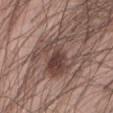Q: Is there a histopathology result?
A: catalogued during a skin exam; not biopsied
Q: Where on the body is the lesion?
A: the abdomen
Q: How was the tile lit?
A: white-light illumination
Q: Patient demographics?
A: male, about 55 years old
Q: How was this image acquired?
A: total-body-photography crop, ~15 mm field of view
Q: Automated lesion metrics?
A: a lesion area of about 18 mm²; an average lesion color of about L≈44 a*≈17 b*≈21 (CIELAB); a border-irregularity rating of about 6.5/10, a color-variation rating of about 8/10, and a peripheral color-asymmetry measure near 3; an automated nevus-likeness rating near 85 out of 100 and a lesion-detection confidence of about 100/100
Q: Lesion size?
A: ~6.5 mm (longest diameter)